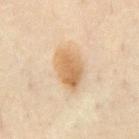| feature | finding |
|---|---|
| automated lesion analysis | an eccentricity of roughly 0.75 and a symmetry-axis asymmetry near 0.2; a mean CIELAB color near L≈65 a*≈16 b*≈38, about 11 CIELAB-L* units darker than the surrounding skin, and a normalized border contrast of about 8; border irregularity of about 2 on a 0–10 scale, a color-variation rating of about 5/10, and a peripheral color-asymmetry measure near 2 |
| subject | male, in their mid-40s |
| image source | total-body-photography crop, ~15 mm field of view |
| anatomic site | the chest |
| lesion diameter | about 5 mm |